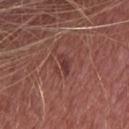Clinical impression:
Part of a total-body skin-imaging series; this lesion was reviewed on a skin check and was not flagged for biopsy.
Acquisition and patient details:
This image is a 15 mm lesion crop taken from a total-body photograph. This is a white-light tile. The lesion's longest dimension is about 2.5 mm. A female subject, approximately 40 years of age. Automated tile analysis of the lesion measured roughly 8 lightness units darker than nearby skin and a normalized lesion–skin contrast near 7. The software also gave an automated nevus-likeness rating near 0 out of 100 and lesion-presence confidence of about 100/100. On the head or neck.